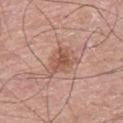Q: Was this lesion biopsied?
A: no biopsy performed (imaged during a skin exam)